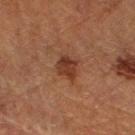– notes · catalogued during a skin exam; not biopsied
– subject · male, roughly 75 years of age
– size · about 2.5 mm
– image-analysis metrics · a mean CIELAB color near L≈29 a*≈19 b*≈25 and a normalized lesion–skin contrast near 8.5; a nevus-likeness score of about 70/100 and a lesion-detection confidence of about 100/100
– site · the right forearm
– imaging modality · ~15 mm tile from a whole-body skin photo